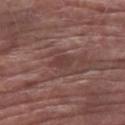<lesion>
<biopsy_status>not biopsied; imaged during a skin examination</biopsy_status>
<automated_metrics>
  <area_mm2_approx>4.0</area_mm2_approx>
  <eccentricity>0.8</eccentricity>
</automated_metrics>
<patient>
  <sex>male</sex>
  <age_approx>80</age_approx>
</patient>
<image>
  <source>total-body photography crop</source>
  <field_of_view_mm>15</field_of_view_mm>
</image>
<site>arm</site>
<lesion_size>
  <long_diameter_mm_approx>2.5</long_diameter_mm_approx>
</lesion_size>
<lighting>white-light</lighting>
</lesion>